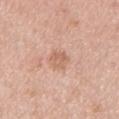Q: Is there a histopathology result?
A: catalogued during a skin exam; not biopsied
Q: What is the lesion's diameter?
A: ~2.5 mm (longest diameter)
Q: Automated lesion metrics?
A: a lesion area of about 4.5 mm², an eccentricity of roughly 0.6, and two-axis asymmetry of about 0.25; a lesion color around L≈63 a*≈21 b*≈31 in CIELAB and a normalized border contrast of about 5.5
Q: What is the imaging modality?
A: total-body-photography crop, ~15 mm field of view
Q: What is the anatomic site?
A: the chest
Q: What are the patient's age and sex?
A: male, in their mid- to late 50s